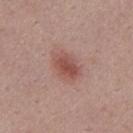Clinical impression:
This lesion was catalogued during total-body skin photography and was not selected for biopsy.
Acquisition and patient details:
The tile uses white-light illumination. About 4 mm across. The subject is a male in their mid-40s. The lesion-visualizer software estimated a footprint of about 8.5 mm², an eccentricity of roughly 0.7, and a symmetry-axis asymmetry near 0.2. The software also gave a within-lesion color-variation index near 4/10 and peripheral color asymmetry of about 1. A close-up tile cropped from a whole-body skin photograph, about 15 mm across. From the back.